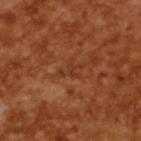Findings:
* notes · imaged on a skin check; not biopsied
* imaging modality · ~15 mm crop, total-body skin-cancer survey
* subject · male, aged 63 to 67